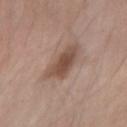notes: imaged on a skin check; not biopsied | patient: male, roughly 25 years of age | automated metrics: a footprint of about 10 mm² and a shape-asymmetry score of about 0.3 (0 = symmetric); a mean CIELAB color near L≈49 a*≈17 b*≈26, about 11 CIELAB-L* units darker than the surrounding skin, and a normalized lesion–skin contrast near 8; a nevus-likeness score of about 80/100 and lesion-presence confidence of about 100/100 | site: the right forearm | image source: ~15 mm tile from a whole-body skin photo.